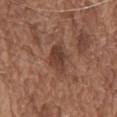biopsy status — imaged on a skin check; not biopsied
location — the chest
image — ~15 mm tile from a whole-body skin photo
lesion diameter — ≈4 mm
patient — male, roughly 75 years of age